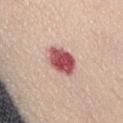workup = no biopsy performed (imaged during a skin exam)
automated lesion analysis = a footprint of about 10 mm², a shape eccentricity near 0.8, and a symmetry-axis asymmetry near 0.15; an average lesion color of about L≈54 a*≈30 b*≈22 (CIELAB), a lesion–skin lightness drop of about 19, and a normalized border contrast of about 12; a nevus-likeness score of about 0/100
image = ~15 mm tile from a whole-body skin photo
anatomic site = the abdomen
subject = female, aged 43 to 47
diameter = ~4.5 mm (longest diameter)